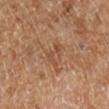{"lighting": "cross-polarized", "patient": {"sex": "female"}, "site": "right lower leg", "automated_metrics": {"vs_skin_darker_L": 7.0, "vs_skin_contrast_norm": 5.0, "nevus_likeness_0_100": 0, "lesion_detection_confidence_0_100": 100}, "image": {"source": "total-body photography crop", "field_of_view_mm": 15}, "lesion_size": {"long_diameter_mm_approx": 3.0}}